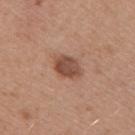biopsy_status: not biopsied; imaged during a skin examination
site: right upper arm
image:
  source: total-body photography crop
  field_of_view_mm: 15
lighting: white-light
automated_metrics:
  area_mm2_approx: 7.0
  eccentricity: 0.7
  shape_asymmetry: 0.15
  cielab_L: 49
  cielab_a: 22
  cielab_b: 28
  vs_skin_darker_L: 12.0
  vs_skin_contrast_norm: 8.5
patient:
  sex: male
  age_approx: 50